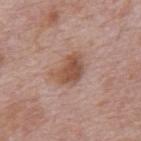Impression: Imaged during a routine full-body skin examination; the lesion was not biopsied and no histopathology is available. Acquisition and patient details: From the mid back. A male patient about 75 years old. A region of skin cropped from a whole-body photographic capture, roughly 15 mm wide.